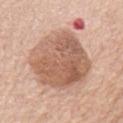Case summary:
• biopsy status · total-body-photography surveillance lesion; no biopsy
• patient · male, aged 78–82
• body site · the front of the torso
• automated lesion analysis · a footprint of about 55 mm², a shape eccentricity near 0.75, and two-axis asymmetry of about 0.25; a nevus-likeness score of about 0/100 and a lesion-detection confidence of about 100/100
• imaging modality · total-body-photography crop, ~15 mm field of view